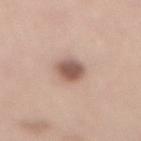Image and clinical context: The lesion is on the mid back. A 15 mm crop from a total-body photograph taken for skin-cancer surveillance. A female subject approximately 50 years of age. Captured under white-light illumination.